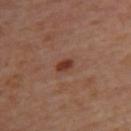Assessment:
Recorded during total-body skin imaging; not selected for excision or biopsy.
Context:
A 15 mm close-up extracted from a 3D total-body photography capture. This is a cross-polarized tile. The total-body-photography lesion software estimated about 10 CIELAB-L* units darker than the surrounding skin. It also reported a border-irregularity rating of about 2/10 and peripheral color asymmetry of about 0.5. The software also gave a nevus-likeness score of about 90/100 and a detector confidence of about 100 out of 100 that the crop contains a lesion. From the upper back. The patient is a female aged approximately 40.